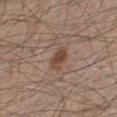The lesion was photographed on a routine skin check and not biopsied; there is no pathology result. Approximately 3 mm at its widest. A male patient approximately 45 years of age. Captured under white-light illumination. The lesion-visualizer software estimated a normalized border contrast of about 7.5. The analysis additionally found a nevus-likeness score of about 90/100 and a detector confidence of about 100 out of 100 that the crop contains a lesion. The lesion is located on the left lower leg. A roughly 15 mm field-of-view crop from a total-body skin photograph.